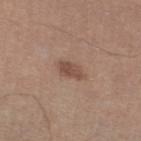Notes:
• follow-up · total-body-photography surveillance lesion; no biopsy
• subject · male, approximately 55 years of age
• acquisition · ~15 mm tile from a whole-body skin photo
• anatomic site · the left lower leg
• tile lighting · white-light